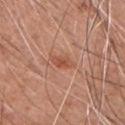The lesion was tiled from a total-body skin photograph and was not biopsied.
On the chest.
Approximately 3 mm at its widest.
Imaged with white-light lighting.
A male subject about 60 years old.
A 15 mm crop from a total-body photograph taken for skin-cancer surveillance.